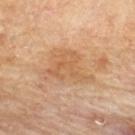<case>
  <biopsy_status>not biopsied; imaged during a skin examination</biopsy_status>
  <patient>
    <sex>male</sex>
    <age_approx>85+</age_approx>
  </patient>
  <site>upper back</site>
  <image>
    <source>total-body photography crop</source>
    <field_of_view_mm>15</field_of_view_mm>
  </image>
</case>